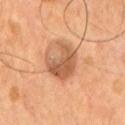Q: What kind of image is this?
A: ~15 mm crop, total-body skin-cancer survey
Q: What did automated image analysis measure?
A: an area of roughly 13 mm², an outline eccentricity of about 0.65 (0 = round, 1 = elongated), and a shape-asymmetry score of about 0.4 (0 = symmetric); a border-irregularity rating of about 4.5/10, a color-variation rating of about 4.5/10, and peripheral color asymmetry of about 1.5; a classifier nevus-likeness of about 35/100 and a detector confidence of about 100 out of 100 that the crop contains a lesion
Q: Where on the body is the lesion?
A: the chest
Q: Illumination type?
A: cross-polarized illumination
Q: What is the lesion's diameter?
A: about 4.5 mm
Q: Who is the patient?
A: male, aged 53 to 57